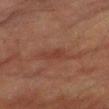workup: total-body-photography surveillance lesion; no biopsy
acquisition: 15 mm crop, total-body photography
automated lesion analysis: a border-irregularity index near 4/10, a color-variation rating of about 0.5/10, and peripheral color asymmetry of about 0; an automated nevus-likeness rating near 0 out of 100
anatomic site: the right thigh
subject: male, in their mid-80s or older